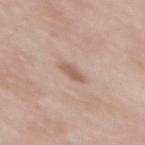Imaged during a routine full-body skin examination; the lesion was not biopsied and no histopathology is available.
About 2.5 mm across.
A roughly 15 mm field-of-view crop from a total-body skin photograph.
The lesion is located on the upper back.
The subject is a female aged approximately 50.